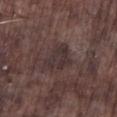follow-up — no biopsy performed (imaged during a skin exam); site — the leg; imaging modality — ~15 mm tile from a whole-body skin photo; subject — male, aged approximately 75.